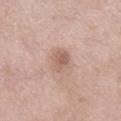Findings:
– follow-up: imaged on a skin check; not biopsied
– automated metrics: an area of roughly 5.5 mm², an eccentricity of roughly 0.6, and a symmetry-axis asymmetry near 0.15
– acquisition: 15 mm crop, total-body photography
– anatomic site: the right lower leg
– tile lighting: white-light illumination
– subject: female, aged 53 to 57